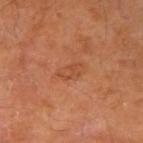workup: total-body-photography surveillance lesion; no biopsy | subject: male, aged 63–67 | lighting: cross-polarized illumination | location: the right upper arm | imaging modality: ~15 mm crop, total-body skin-cancer survey.